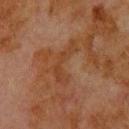Assessment:
Part of a total-body skin-imaging series; this lesion was reviewed on a skin check and was not flagged for biopsy.
Image and clinical context:
This is a cross-polarized tile. About 5.5 mm across. On the upper back. This image is a 15 mm lesion crop taken from a total-body photograph. A male patient, aged 78–82.